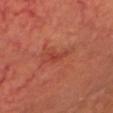notes: no biopsy performed (imaged during a skin exam) | imaging modality: 15 mm crop, total-body photography | patient: male, approximately 65 years of age | location: the head or neck | lesion diameter: about 3 mm | TBP lesion metrics: about 7 CIELAB-L* units darker than the surrounding skin and a lesion-to-skin contrast of about 5.5 (normalized; higher = more distinct); a border-irregularity rating of about 5.5/10 and internal color variation of about 0.5 on a 0–10 scale; a detector confidence of about 95 out of 100 that the crop contains a lesion | lighting: cross-polarized illumination.